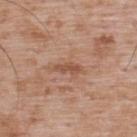The lesion was tiled from a total-body skin photograph and was not biopsied. The subject is a male about 50 years old. A close-up tile cropped from a whole-body skin photograph, about 15 mm across. Captured under white-light illumination. From the back. Approximately 3 mm at its widest.